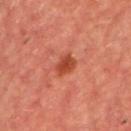{"patient": {"sex": "male", "age_approx": 60}, "site": "chest", "lesion_size": {"long_diameter_mm_approx": 2.5}, "image": {"source": "total-body photography crop", "field_of_view_mm": 15}}